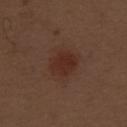acquisition = ~15 mm crop, total-body skin-cancer survey
patient = male, approximately 70 years of age
illumination = white-light
anatomic site = the right thigh
size = ≈3.5 mm